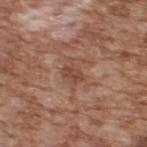This lesion was catalogued during total-body skin photography and was not selected for biopsy. A region of skin cropped from a whole-body photographic capture, roughly 15 mm wide. The lesion is located on the back. The patient is a male aged 63–67.